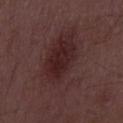image-analysis metrics = an average lesion color of about L≈26 a*≈19 b*≈16 (CIELAB), about 7 CIELAB-L* units darker than the surrounding skin, and a normalized border contrast of about 7; a border-irregularity index near 5.5/10 and a peripheral color-asymmetry measure near 1.5 | image = total-body-photography crop, ~15 mm field of view | size = ≈9.5 mm | patient = male, aged approximately 50 | illumination = white-light.